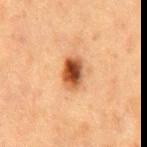Q: Was a biopsy performed?
A: no biopsy performed (imaged during a skin exam)
Q: Where on the body is the lesion?
A: the mid back
Q: What is the imaging modality?
A: ~15 mm crop, total-body skin-cancer survey
Q: How was the tile lit?
A: cross-polarized
Q: Who is the patient?
A: male, aged around 75
Q: What did automated image analysis measure?
A: a border-irregularity index near 1.5/10 and a peripheral color-asymmetry measure near 2; an automated nevus-likeness rating near 100 out of 100 and a detector confidence of about 100 out of 100 that the crop contains a lesion
Q: How large is the lesion?
A: ≈3.5 mm